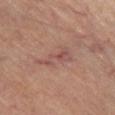| field | value |
|---|---|
| patient | male, aged approximately 60 |
| imaging modality | total-body-photography crop, ~15 mm field of view |
| site | the right lower leg |
| lesion diameter | about 5 mm |